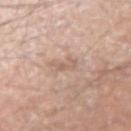No biopsy was performed on this lesion — it was imaged during a full skin examination and was not determined to be concerning.
The lesion is located on the right upper arm.
A region of skin cropped from a whole-body photographic capture, roughly 15 mm wide.
A male subject about 55 years old.
Automated image analysis of the tile measured an average lesion color of about L≈61 a*≈17 b*≈27 (CIELAB), roughly 8 lightness units darker than nearby skin, and a normalized border contrast of about 5. And it measured border irregularity of about 6 on a 0–10 scale and internal color variation of about 0 on a 0–10 scale. And it measured a lesion-detection confidence of about 100/100.